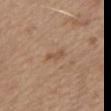biopsy status: catalogued during a skin exam; not biopsied
image-analysis metrics: about 8 CIELAB-L* units darker than the surrounding skin; a border-irregularity rating of about 4.5/10, internal color variation of about 0 on a 0–10 scale, and a peripheral color-asymmetry measure near 0; a classifier nevus-likeness of about 0/100
lesion size: about 2.5 mm
imaging modality: total-body-photography crop, ~15 mm field of view
illumination: white-light illumination
subject: male, aged 68 to 72
anatomic site: the mid back